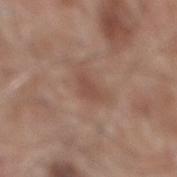<case>
<biopsy_status>not biopsied; imaged during a skin examination</biopsy_status>
<patient>
  <sex>male</sex>
  <age_approx>60</age_approx>
</patient>
<site>mid back</site>
<automated_metrics>
  <area_mm2_approx>3.0</area_mm2_approx>
  <eccentricity>0.85</eccentricity>
  <cielab_L>47</cielab_L>
  <cielab_a>21</cielab_a>
  <cielab_b>25</cielab_b>
  <vs_skin_darker_L>7.0</vs_skin_darker_L>
  <vs_skin_contrast_norm>5.5</vs_skin_contrast_norm>
  <nevus_likeness_0_100>0</nevus_likeness_0_100>
  <lesion_detection_confidence_0_100>100</lesion_detection_confidence_0_100>
</automated_metrics>
<lesion_size>
  <long_diameter_mm_approx>2.5</long_diameter_mm_approx>
</lesion_size>
<image>
  <source>total-body photography crop</source>
  <field_of_view_mm>15</field_of_view_mm>
</image>
<lighting>white-light</lighting>
</case>